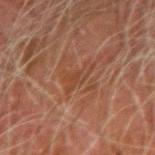Clinical impression:
The lesion was photographed on a routine skin check and not biopsied; there is no pathology result.